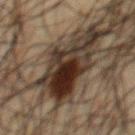<tbp_lesion>
<biopsy_status>not biopsied; imaged during a skin examination</biopsy_status>
</tbp_lesion>